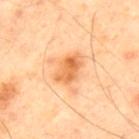Recorded during total-body skin imaging; not selected for excision or biopsy.
The patient is a male in their 40s.
A close-up tile cropped from a whole-body skin photograph, about 15 mm across.
An algorithmic analysis of the crop reported a lesion area of about 8 mm², a shape eccentricity near 0.75, and a shape-asymmetry score of about 0.25 (0 = symmetric). It also reported a lesion color around L≈70 a*≈28 b*≈47 in CIELAB. The software also gave peripheral color asymmetry of about 2.
This is a cross-polarized tile.
The lesion's longest dimension is about 4 mm.
Located on the upper back.